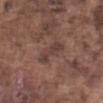Findings:
– lighting — white-light
– patient — male, in their mid-70s
– image — ~15 mm tile from a whole-body skin photo
– body site — the front of the torso
– diameter — ≈4 mm